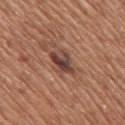The lesion was photographed on a routine skin check and not biopsied; there is no pathology result.
A close-up tile cropped from a whole-body skin photograph, about 15 mm across.
The lesion is located on the mid back.
Automated image analysis of the tile measured an area of roughly 7 mm², an eccentricity of roughly 0.75, and a shape-asymmetry score of about 0.25 (0 = symmetric). The software also gave a border-irregularity index near 3/10.
About 3.5 mm across.
Captured under white-light illumination.
The patient is a male roughly 65 years of age.